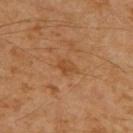Q: Was this lesion biopsied?
A: imaged on a skin check; not biopsied
Q: What is the anatomic site?
A: the upper back
Q: What are the patient's age and sex?
A: male, aged 58 to 62
Q: How was this image acquired?
A: ~15 mm tile from a whole-body skin photo